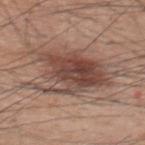Imaged during a routine full-body skin examination; the lesion was not biopsied and no histopathology is available.
A male patient, aged around 60.
The lesion is on the upper back.
A region of skin cropped from a whole-body photographic capture, roughly 15 mm wide.
About 9 mm across.
Captured under white-light illumination.